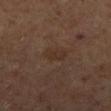Assessment:
The lesion was tiled from a total-body skin photograph and was not biopsied.
Background:
A male patient, aged around 65. A 15 mm close-up extracted from a 3D total-body photography capture. On the right lower leg.